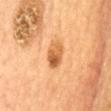Assessment:
Recorded during total-body skin imaging; not selected for excision or biopsy.
Background:
A 15 mm close-up tile from a total-body photography series done for melanoma screening. The recorded lesion diameter is about 3.5 mm. The subject is a female aged approximately 60. Captured under cross-polarized illumination. On the chest.